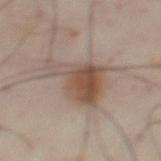biopsy status: no biopsy performed (imaged during a skin exam); subject: male, in their mid-50s; TBP lesion metrics: a shape eccentricity near 0.8 and a symmetry-axis asymmetry near 0.5; body site: the abdomen; image source: 15 mm crop, total-body photography; size: about 7 mm.